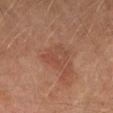No biopsy was performed on this lesion — it was imaged during a full skin examination and was not determined to be concerning. The subject is a male about 75 years old. Automated image analysis of the tile measured a footprint of about 8 mm² and an eccentricity of roughly 0.75. The analysis additionally found an average lesion color of about L≈38 a*≈19 b*≈25 (CIELAB), a lesion–skin lightness drop of about 5, and a lesion-to-skin contrast of about 4.5 (normalized; higher = more distinct). The analysis additionally found border irregularity of about 3.5 on a 0–10 scale, internal color variation of about 3.5 on a 0–10 scale, and peripheral color asymmetry of about 1. It also reported a nevus-likeness score of about 0/100 and lesion-presence confidence of about 100/100. A 15 mm crop from a total-body photograph taken for skin-cancer surveillance. Approximately 4 mm at its widest. From the right thigh. Captured under cross-polarized illumination.